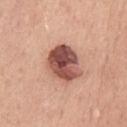Case summary:
- notes — imaged on a skin check; not biopsied
- acquisition — ~15 mm tile from a whole-body skin photo
- patient — male, in their 50s
- location — the chest
- diameter — ~5 mm (longest diameter)
- automated metrics — an average lesion color of about L≈51 a*≈25 b*≈26 (CIELAB), roughly 19 lightness units darker than nearby skin, and a normalized border contrast of about 12; border irregularity of about 2 on a 0–10 scale, a color-variation rating of about 9/10, and a peripheral color-asymmetry measure near 3.5; a nevus-likeness score of about 25/100 and a detector confidence of about 100 out of 100 that the crop contains a lesion
- tile lighting — white-light